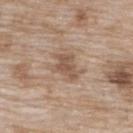The lesion was tiled from a total-body skin photograph and was not biopsied.
The recorded lesion diameter is about 4 mm.
A female subject, aged 73 to 77.
Cropped from a total-body skin-imaging series; the visible field is about 15 mm.
Captured under white-light illumination.
Automated image analysis of the tile measured a nevus-likeness score of about 5/100 and lesion-presence confidence of about 100/100.
The lesion is located on the upper back.